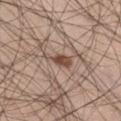Impression: This lesion was catalogued during total-body skin photography and was not selected for biopsy. Clinical summary: A lesion tile, about 15 mm wide, cut from a 3D total-body photograph. A male subject aged 28 to 32. This is a white-light tile. The lesion is located on the left thigh. Longest diameter approximately 3.5 mm. The total-body-photography lesion software estimated a nevus-likeness score of about 100/100 and lesion-presence confidence of about 100/100.